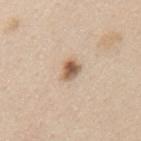The lesion was tiled from a total-body skin photograph and was not biopsied. About 2.5 mm across. The subject is a female roughly 45 years of age. On the back. Imaged with white-light lighting. The total-body-photography lesion software estimated an eccentricity of roughly 0.65 and a shape-asymmetry score of about 0.25 (0 = symmetric). It also reported about 15 CIELAB-L* units darker than the surrounding skin. A close-up tile cropped from a whole-body skin photograph, about 15 mm across.